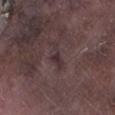notes: imaged on a skin check; not biopsied
lesion size: ~3.5 mm (longest diameter)
anatomic site: the left lower leg
acquisition: 15 mm crop, total-body photography
subject: male, in their mid-70s
tile lighting: white-light illumination